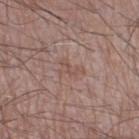<lesion>
<patient>
  <sex>male</sex>
  <age_approx>50</age_approx>
</patient>
<site>right thigh</site>
<lighting>white-light</lighting>
<automated_metrics>
  <eccentricity>0.75</eccentricity>
  <shape_asymmetry>0.5</shape_asymmetry>
  <cielab_L>51</cielab_L>
  <cielab_a>18</cielab_a>
  <cielab_b>24</cielab_b>
  <color_variation_0_10>0.0</color_variation_0_10>
  <peripheral_color_asymmetry>0.0</peripheral_color_asymmetry>
  <nevus_likeness_0_100>0</nevus_likeness_0_100>
  <lesion_detection_confidence_0_100>95</lesion_detection_confidence_0_100>
</automated_metrics>
<image>
  <source>total-body photography crop</source>
  <field_of_view_mm>15</field_of_view_mm>
</image>
</lesion>